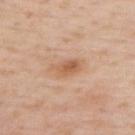Assessment: Captured during whole-body skin photography for melanoma surveillance; the lesion was not biopsied. Acquisition and patient details: The tile uses white-light illumination. Located on the upper back. About 4.5 mm across. Cropped from a whole-body photographic skin survey; the tile spans about 15 mm. The subject is a male in their mid- to late 70s.